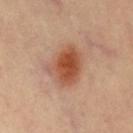Assessment:
The lesion was photographed on a routine skin check and not biopsied; there is no pathology result.
Clinical summary:
Imaged with cross-polarized lighting. Longest diameter approximately 5 mm. Cropped from a whole-body photographic skin survey; the tile spans about 15 mm. Located on the chest.